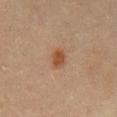Part of a total-body skin-imaging series; this lesion was reviewed on a skin check and was not flagged for biopsy. The tile uses cross-polarized illumination. About 3 mm across. The patient is a male aged around 45. Located on the chest. Cropped from a total-body skin-imaging series; the visible field is about 15 mm.